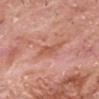notes: total-body-photography surveillance lesion; no biopsy | lesion size: ≈3 mm | anatomic site: the head or neck | automated metrics: an area of roughly 4 mm², an eccentricity of roughly 0.85, and two-axis asymmetry of about 0.35; a lesion color around L≈56 a*≈27 b*≈31 in CIELAB and roughly 8 lightness units darker than nearby skin; a nevus-likeness score of about 0/100 and lesion-presence confidence of about 100/100 | image: 15 mm crop, total-body photography | illumination: white-light illumination | patient: male, roughly 80 years of age.